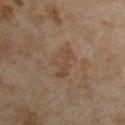• image source · 15 mm crop, total-body photography
• patient · male, aged approximately 55
• automated metrics · a within-lesion color-variation index near 2.5/10 and radial color variation of about 1; an automated nevus-likeness rating near 0 out of 100 and lesion-presence confidence of about 100/100
• location · the right upper arm
• illumination · cross-polarized illumination
• lesion size · about 4.5 mm